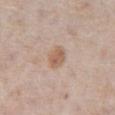No biopsy was performed on this lesion — it was imaged during a full skin examination and was not determined to be concerning. On the front of the torso. A male patient in their mid- to late 70s. Imaged with white-light lighting. A lesion tile, about 15 mm wide, cut from a 3D total-body photograph.